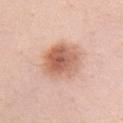• follow-up: imaged on a skin check; not biopsied
• tile lighting: white-light illumination
• subject: female, aged 38–42
• TBP lesion metrics: an area of roughly 17 mm², a shape eccentricity near 0.45, and two-axis asymmetry of about 0.15; an automated nevus-likeness rating near 95 out of 100 and a lesion-detection confidence of about 100/100
• anatomic site: the chest
• diameter: ~5 mm (longest diameter)
• image: total-body-photography crop, ~15 mm field of view